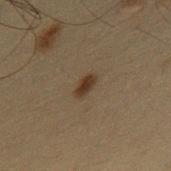lesion diameter=about 2.5 mm; image-analysis metrics=an automated nevus-likeness rating near 95 out of 100 and a detector confidence of about 100 out of 100 that the crop contains a lesion; site=the mid back; lighting=cross-polarized; subject=male, roughly 65 years of age; acquisition=15 mm crop, total-body photography.